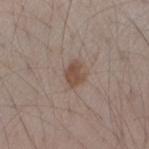Q: Was this lesion biopsied?
A: total-body-photography surveillance lesion; no biopsy
Q: What is the imaging modality?
A: ~15 mm crop, total-body skin-cancer survey
Q: What is the anatomic site?
A: the right upper arm
Q: What are the patient's age and sex?
A: male, aged 53 to 57
Q: How large is the lesion?
A: about 2.5 mm